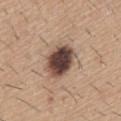Impression:
Imaged during a routine full-body skin examination; the lesion was not biopsied and no histopathology is available.
Context:
On the abdomen. A close-up tile cropped from a whole-body skin photograph, about 15 mm across. A male patient aged 58–62.